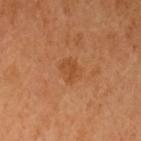biopsy status = catalogued during a skin exam; not biopsied | illumination = cross-polarized | patient = female, roughly 55 years of age | diameter = ≈3 mm | image source = ~15 mm crop, total-body skin-cancer survey | location = the left upper arm | automated metrics = roughly 6 lightness units darker than nearby skin; border irregularity of about 1.5 on a 0–10 scale and a color-variation rating of about 2.5/10.